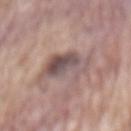Captured during whole-body skin photography for melanoma surveillance; the lesion was not biopsied. On the abdomen. The subject is a male aged approximately 60. A region of skin cropped from a whole-body photographic capture, roughly 15 mm wide.